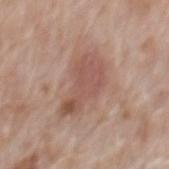Assessment: Imaged during a routine full-body skin examination; the lesion was not biopsied and no histopathology is available. Background: This image is a 15 mm lesion crop taken from a total-body photograph. The tile uses white-light illumination. Automated tile analysis of the lesion measured a shape eccentricity near 0.85 and a symmetry-axis asymmetry near 0.4. The analysis additionally found a mean CIELAB color near L≈53 a*≈21 b*≈25, about 9 CIELAB-L* units darker than the surrounding skin, and a normalized lesion–skin contrast near 6.5. The lesion is on the mid back. A male subject, aged around 70.